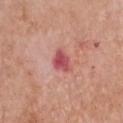{
  "biopsy_status": "not biopsied; imaged during a skin examination"
}